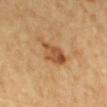follow-up — catalogued during a skin exam; not biopsied | acquisition — ~15 mm tile from a whole-body skin photo | anatomic site — the mid back | TBP lesion metrics — a lesion area of about 7.5 mm², an eccentricity of roughly 0.85, and a symmetry-axis asymmetry near 0.3; an automated nevus-likeness rating near 25 out of 100 and a detector confidence of about 100 out of 100 that the crop contains a lesion | illumination — cross-polarized illumination | patient — male, in their mid- to late 60s.